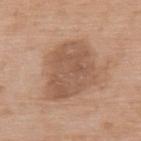No biopsy was performed on this lesion — it was imaged during a full skin examination and was not determined to be concerning.
The tile uses white-light illumination.
Approximately 7 mm at its widest.
The lesion is on the upper back.
The subject is a female aged approximately 75.
A region of skin cropped from a whole-body photographic capture, roughly 15 mm wide.